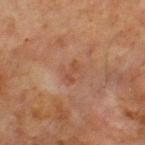Impression:
No biopsy was performed on this lesion — it was imaged during a full skin examination and was not determined to be concerning.
Context:
This is a cross-polarized tile. Longest diameter approximately 2.5 mm. On the chest. A male patient in their mid- to late 60s. Cropped from a total-body skin-imaging series; the visible field is about 15 mm. An algorithmic analysis of the crop reported border irregularity of about 6.5 on a 0–10 scale, a color-variation rating of about 0/10, and peripheral color asymmetry of about 0. The software also gave a lesion-detection confidence of about 100/100.